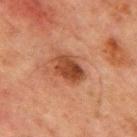Part of a total-body skin-imaging series; this lesion was reviewed on a skin check and was not flagged for biopsy. The subject is a male aged 63 to 67. On the chest. A region of skin cropped from a whole-body photographic capture, roughly 15 mm wide.